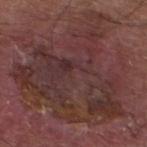biopsy status: catalogued during a skin exam; not biopsied
image source: ~15 mm tile from a whole-body skin photo
patient: male, aged 78–82
TBP lesion metrics: a lesion area of about 100 mm² and a shape-asymmetry score of about 0.35 (0 = symmetric); a lesion-to-skin contrast of about 6.5 (normalized; higher = more distinct); border irregularity of about 7.5 on a 0–10 scale and a peripheral color-asymmetry measure near 2; a nevus-likeness score of about 0/100 and lesion-presence confidence of about 90/100
anatomic site: the left lower leg